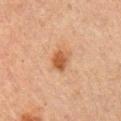Captured during whole-body skin photography for melanoma surveillance; the lesion was not biopsied.
Approximately 3 mm at its widest.
Located on the right upper arm.
Automated tile analysis of the lesion measured a footprint of about 5.5 mm² and an outline eccentricity of about 0.65 (0 = round, 1 = elongated).
A male patient, about 50 years old.
Imaged with cross-polarized lighting.
A close-up tile cropped from a whole-body skin photograph, about 15 mm across.